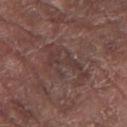Case summary:
- workup · imaged on a skin check; not biopsied
- subject · male, aged 78–82
- diameter · ~6.5 mm (longest diameter)
- TBP lesion metrics · an area of roughly 13 mm², an eccentricity of roughly 0.85, and a symmetry-axis asymmetry near 0.55; a lesion color around L≈37 a*≈18 b*≈19 in CIELAB, a lesion–skin lightness drop of about 6, and a normalized border contrast of about 5; border irregularity of about 9 on a 0–10 scale and a peripheral color-asymmetry measure near 1; a detector confidence of about 60 out of 100 that the crop contains a lesion
- location · the right forearm
- acquisition · total-body-photography crop, ~15 mm field of view